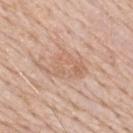The lesion was tiled from a total-body skin photograph and was not biopsied.
A 15 mm close-up extracted from a 3D total-body photography capture.
From the mid back.
A male patient, about 80 years old.